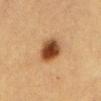follow-up: imaged on a skin check; not biopsied | site: the front of the torso | patient: female, in their mid-50s | imaging modality: ~15 mm tile from a whole-body skin photo | lesion diameter: ~3.5 mm (longest diameter) | lighting: cross-polarized.